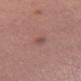Findings:
* workup: no biopsy performed (imaged during a skin exam)
* tile lighting: white-light
* lesion diameter: ≈2.5 mm
* imaging modality: 15 mm crop, total-body photography
* patient: female, aged 48–52
* body site: the right thigh